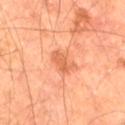Automated image analysis of the tile measured a lesion area of about 5 mm², an eccentricity of roughly 0.75, and two-axis asymmetry of about 0.3. It also reported a nevus-likeness score of about 5/100. A lesion tile, about 15 mm wide, cut from a 3D total-body photograph. The recorded lesion diameter is about 3 mm. The subject is a male roughly 65 years of age. The tile uses cross-polarized illumination. The lesion is located on the right thigh.